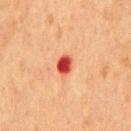{
  "biopsy_status": "not biopsied; imaged during a skin examination",
  "lesion_size": {
    "long_diameter_mm_approx": 2.5
  },
  "image": {
    "source": "total-body photography crop",
    "field_of_view_mm": 15
  },
  "lighting": "cross-polarized",
  "patient": {
    "sex": "male",
    "age_approx": 65
  },
  "site": "chest"
}